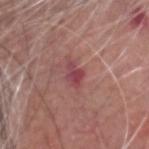Impression:
The lesion was photographed on a routine skin check and not biopsied; there is no pathology result.
Context:
About 3 mm across. A male patient, aged 63 to 67. This is a white-light tile. Automated image analysis of the tile measured a border-irregularity index near 2.5/10 and internal color variation of about 3 on a 0–10 scale. This image is a 15 mm lesion crop taken from a total-body photograph. From the head or neck.